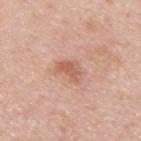Notes:
* workup — imaged on a skin check; not biopsied
* lesion diameter — ≈3 mm
* patient — male, aged approximately 45
* lighting — white-light illumination
* anatomic site — the upper back
* TBP lesion metrics — an area of roughly 4.5 mm², an eccentricity of roughly 0.75, and a symmetry-axis asymmetry near 0.4
* image source — 15 mm crop, total-body photography